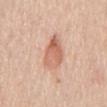Recorded during total-body skin imaging; not selected for excision or biopsy. From the front of the torso. The lesion-visualizer software estimated a footprint of about 10 mm² and two-axis asymmetry of about 0.25. The software also gave a border-irregularity index near 2.5/10, a color-variation rating of about 6.5/10, and peripheral color asymmetry of about 2.5. A roughly 15 mm field-of-view crop from a total-body skin photograph. A female patient, aged 63 to 67. Captured under white-light illumination. Measured at roughly 5 mm in maximum diameter.